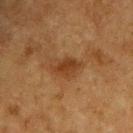{
  "lighting": "cross-polarized",
  "patient": {
    "sex": "male",
    "age_approx": 75
  },
  "site": "upper back",
  "image": {
    "source": "total-body photography crop",
    "field_of_view_mm": 15
  },
  "lesion_size": {
    "long_diameter_mm_approx": 3.0
  },
  "automated_metrics": {
    "area_mm2_approx": 5.0,
    "eccentricity": 0.75,
    "cielab_L": 31,
    "cielab_a": 19,
    "cielab_b": 30,
    "vs_skin_contrast_norm": 7.5,
    "nevus_likeness_0_100": 55,
    "lesion_detection_confidence_0_100": 100
  }
}